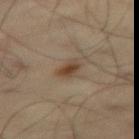The lesion was tiled from a total-body skin photograph and was not biopsied.
Imaged with cross-polarized lighting.
The lesion's longest dimension is about 2.5 mm.
A lesion tile, about 15 mm wide, cut from a 3D total-body photograph.
The lesion is located on the right thigh.
A male subject roughly 55 years of age.